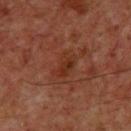Clinical impression:
Recorded during total-body skin imaging; not selected for excision or biopsy.
Background:
Captured under cross-polarized illumination. Located on the chest. About 3 mm across. The subject is a male approximately 60 years of age. A roughly 15 mm field-of-view crop from a total-body skin photograph.